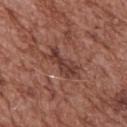biopsy status: imaged on a skin check; not biopsied | lighting: white-light illumination | image source: ~15 mm crop, total-body skin-cancer survey | automated metrics: a footprint of about 8 mm², an outline eccentricity of about 0.9 (0 = round, 1 = elongated), and a symmetry-axis asymmetry near 0.35; border irregularity of about 5.5 on a 0–10 scale, a color-variation rating of about 3/10, and a peripheral color-asymmetry measure near 1; a nevus-likeness score of about 0/100 and lesion-presence confidence of about 95/100 | body site: the upper back | patient: male, approximately 75 years of age | size: about 4.5 mm.